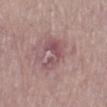{"biopsy_status": "not biopsied; imaged during a skin examination", "image": {"source": "total-body photography crop", "field_of_view_mm": 15}, "automated_metrics": {"border_irregularity_0_10": 6.0, "color_variation_0_10": 4.5, "peripheral_color_asymmetry": 1.5}, "site": "left lower leg", "lesion_size": {"long_diameter_mm_approx": 4.0}, "patient": {"sex": "female", "age_approx": 70}}